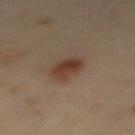{
  "biopsy_status": "not biopsied; imaged during a skin examination",
  "lighting": "cross-polarized",
  "patient": {
    "sex": "male",
    "age_approx": 65
  },
  "site": "mid back",
  "image": {
    "source": "total-body photography crop",
    "field_of_view_mm": 15
  },
  "automated_metrics": {
    "lesion_detection_confidence_0_100": 100
  },
  "lesion_size": {
    "long_diameter_mm_approx": 4.0
  }
}